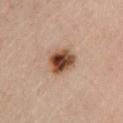Impression:
Imaged during a routine full-body skin examination; the lesion was not biopsied and no histopathology is available.
Acquisition and patient details:
The recorded lesion diameter is about 3.5 mm. Cropped from a whole-body photographic skin survey; the tile spans about 15 mm. A male patient aged 48–52. This is a cross-polarized tile. Located on the left lower leg. The lesion-visualizer software estimated a lesion area of about 9 mm², an eccentricity of roughly 0.55, and a symmetry-axis asymmetry near 0.2. And it measured a border-irregularity index near 2/10, a color-variation rating of about 8/10, and radial color variation of about 2.5.